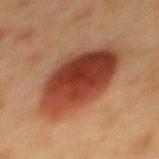The lesion was tiled from a total-body skin photograph and was not biopsied.
The lesion is on the mid back.
A 15 mm crop from a total-body photograph taken for skin-cancer surveillance.
A female subject aged around 40.